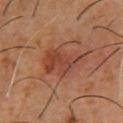| field | value |
|---|---|
| workup | imaged on a skin check; not biopsied |
| body site | the front of the torso |
| patient | male, approximately 55 years of age |
| imaging modality | ~15 mm crop, total-body skin-cancer survey |
| lighting | cross-polarized |
| lesion diameter | about 5.5 mm |